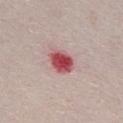Case summary:
– workup — total-body-photography surveillance lesion; no biopsy
– lesion size — ≈3.5 mm
– acquisition — 15 mm crop, total-body photography
– patient — female, roughly 50 years of age
– site — the chest
– tile lighting — white-light
– automated metrics — an area of roughly 8 mm², a shape eccentricity near 0.65, and a symmetry-axis asymmetry near 0.15